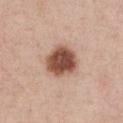Recorded during total-body skin imaging; not selected for excision or biopsy.
A female subject aged around 40.
A 15 mm close-up extracted from a 3D total-body photography capture.
Approximately 4 mm at its widest.
This is a white-light tile.
Located on the chest.
An algorithmic analysis of the crop reported an automated nevus-likeness rating near 95 out of 100 and lesion-presence confidence of about 100/100.